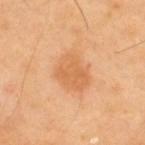The lesion was tiled from a total-body skin photograph and was not biopsied. A close-up tile cropped from a whole-body skin photograph, about 15 mm across. A male patient, aged around 40. Captured under cross-polarized illumination. From the upper back. The recorded lesion diameter is about 4.5 mm.